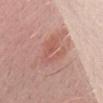Recorded during total-body skin imaging; not selected for excision or biopsy.
From the head or neck.
The recorded lesion diameter is about 4 mm.
Automated image analysis of the tile measured a border-irregularity rating of about 7.5/10.
A region of skin cropped from a whole-body photographic capture, roughly 15 mm wide.
A male patient, aged approximately 25.